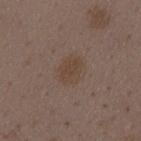Imaged during a routine full-body skin examination; the lesion was not biopsied and no histopathology is available. From the mid back. Measured at roughly 3 mm in maximum diameter. Cropped from a whole-body photographic skin survey; the tile spans about 15 mm. Imaged with white-light lighting. An algorithmic analysis of the crop reported a footprint of about 6.5 mm², an eccentricity of roughly 0.65, and a shape-asymmetry score of about 0.2 (0 = symmetric). A female subject approximately 35 years of age.